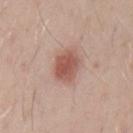Notes:
* notes — imaged on a skin check; not biopsied
* acquisition — ~15 mm crop, total-body skin-cancer survey
* subject — male, aged around 30
* body site — the left upper arm